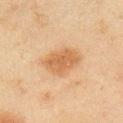follow-up = imaged on a skin check; not biopsied | body site = the arm | image = ~15 mm tile from a whole-body skin photo | subject = male, aged 43–47 | diameter = about 5 mm | lighting = cross-polarized illumination.